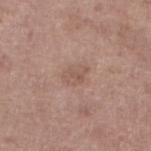Assessment: Recorded during total-body skin imaging; not selected for excision or biopsy. Image and clinical context: An algorithmic analysis of the crop reported a mean CIELAB color near L≈54 a*≈18 b*≈25, a lesion–skin lightness drop of about 7, and a normalized border contrast of about 5. The analysis additionally found a detector confidence of about 100 out of 100 that the crop contains a lesion. A 15 mm close-up tile from a total-body photography series done for melanoma screening. The lesion is on the left lower leg. The patient is a male in their mid-60s. The recorded lesion diameter is about 3 mm. The tile uses white-light illumination.